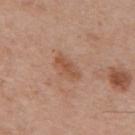biopsy_status: not biopsied; imaged during a skin examination
image:
  source: total-body photography crop
  field_of_view_mm: 15
patient:
  sex: male
  age_approx: 55
site: upper back